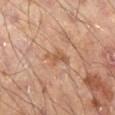{"automated_metrics": {"cielab_L": 52, "cielab_a": 19, "cielab_b": 31, "vs_skin_darker_L": 7.0, "vs_skin_contrast_norm": 5.5, "border_irregularity_0_10": 4.5, "color_variation_0_10": 1.5, "peripheral_color_asymmetry": 0.5}, "patient": {"sex": "male", "age_approx": 55}, "lesion_size": {"long_diameter_mm_approx": 3.5}, "site": "leg", "lighting": "cross-polarized", "image": {"source": "total-body photography crop", "field_of_view_mm": 15}}